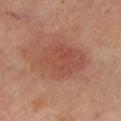Part of a total-body skin-imaging series; this lesion was reviewed on a skin check and was not flagged for biopsy. On the left lower leg. The patient is a male roughly 65 years of age. About 6 mm across. The lesion-visualizer software estimated a border-irregularity index near 2/10 and a within-lesion color-variation index near 3.5/10. A region of skin cropped from a whole-body photographic capture, roughly 15 mm wide. Imaged with cross-polarized lighting.